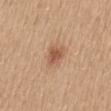Notes:
– follow-up: no biopsy performed (imaged during a skin exam)
– subject: female, in their mid- to late 50s
– location: the mid back
– lesion size: ≈3 mm
– acquisition: ~15 mm tile from a whole-body skin photo
– lighting: white-light illumination